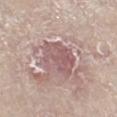Recorded during total-body skin imaging; not selected for excision or biopsy. Cropped from a whole-body photographic skin survey; the tile spans about 15 mm. A female subject aged around 70. From the left lower leg.